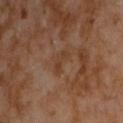Case summary:
• notes: no biopsy performed (imaged during a skin exam)
• acquisition: total-body-photography crop, ~15 mm field of view
• site: the chest
• lighting: cross-polarized
• patient: male, roughly 60 years of age
• diameter: about 3 mm
• TBP lesion metrics: an area of roughly 3 mm², an eccentricity of roughly 0.9, and a shape-asymmetry score of about 0.5 (0 = symmetric); border irregularity of about 5.5 on a 0–10 scale and a within-lesion color-variation index near 0/10; a detector confidence of about 100 out of 100 that the crop contains a lesion